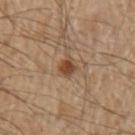| key | value |
|---|---|
| acquisition | ~15 mm tile from a whole-body skin photo |
| site | the arm |
| subject | male, aged approximately 55 |